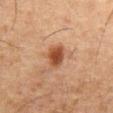Part of a total-body skin-imaging series; this lesion was reviewed on a skin check and was not flagged for biopsy. The total-body-photography lesion software estimated a mean CIELAB color near L≈39 a*≈21 b*≈30, a lesion–skin lightness drop of about 11, and a normalized lesion–skin contrast near 9.5. The tile uses cross-polarized illumination. A close-up tile cropped from a whole-body skin photograph, about 15 mm across. A male patient, roughly 75 years of age. On the abdomen.